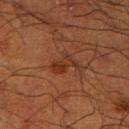Imaged during a routine full-body skin examination; the lesion was not biopsied and no histopathology is available.
A lesion tile, about 15 mm wide, cut from a 3D total-body photograph.
On the right upper arm.
A male patient, roughly 60 years of age.